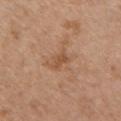- follow-up · imaged on a skin check; not biopsied
- image source · ~15 mm crop, total-body skin-cancer survey
- automated lesion analysis · an eccentricity of roughly 0.8 and a symmetry-axis asymmetry near 0.35; a mean CIELAB color near L≈52 a*≈21 b*≈33 and a lesion–skin lightness drop of about 8; a border-irregularity rating of about 3.5/10 and internal color variation of about 1.5 on a 0–10 scale
- anatomic site · the chest
- diameter · about 2.5 mm
- subject · male, aged 48 to 52
- tile lighting · white-light illumination